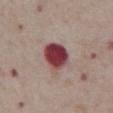Q: Was this lesion biopsied?
A: catalogued during a skin exam; not biopsied
Q: Automated lesion metrics?
A: a lesion area of about 10 mm², a shape eccentricity near 0.5, and two-axis asymmetry of about 0.2; a mean CIELAB color near L≈41 a*≈30 b*≈18, a lesion–skin lightness drop of about 20, and a normalized border contrast of about 15; a within-lesion color-variation index near 4.5/10 and a peripheral color-asymmetry measure near 1.5
Q: Lesion size?
A: about 4 mm
Q: Where on the body is the lesion?
A: the abdomen
Q: What lighting was used for the tile?
A: white-light illumination
Q: Patient demographics?
A: male, about 75 years old
Q: What is the imaging modality?
A: 15 mm crop, total-body photography